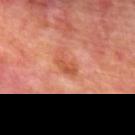* image-analysis metrics · an automated nevus-likeness rating near 0 out of 100 and a lesion-detection confidence of about 100/100
* patient · male, aged approximately 70
* diameter · ≈3 mm
* lighting · cross-polarized
* location · the back
* imaging modality · 15 mm crop, total-body photography